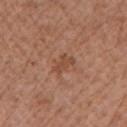The lesion was photographed on a routine skin check and not biopsied; there is no pathology result.
The lesion is on the left upper arm.
The subject is a female in their mid- to late 70s.
A region of skin cropped from a whole-body photographic capture, roughly 15 mm wide.
The lesion's longest dimension is about 3 mm.
The tile uses white-light illumination.